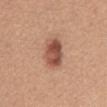{"lesion_size": {"long_diameter_mm_approx": 3.5}, "image": {"source": "total-body photography crop", "field_of_view_mm": 15}, "patient": {"sex": "female", "age_approx": 55}, "site": "abdomen"}